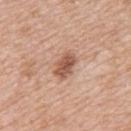biopsy status: imaged on a skin check; not biopsied
site: the back
patient: female, roughly 45 years of age
acquisition: total-body-photography crop, ~15 mm field of view
illumination: white-light
diameter: ≈3.5 mm